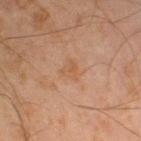The lesion was tiled from a total-body skin photograph and was not biopsied. The lesion is located on the left lower leg. Cropped from a total-body skin-imaging series; the visible field is about 15 mm. Captured under cross-polarized illumination. A male subject, aged approximately 45.